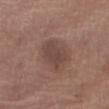Impression:
The lesion was tiled from a total-body skin photograph and was not biopsied.
Background:
This is a white-light tile. A female subject roughly 65 years of age. Cropped from a whole-body photographic skin survey; the tile spans about 15 mm. From the right thigh.